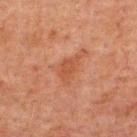Clinical impression: No biopsy was performed on this lesion — it was imaged during a full skin examination and was not determined to be concerning. Context: The lesion is on the upper back. A 15 mm close-up tile from a total-body photography series done for melanoma screening. A male patient approximately 60 years of age.